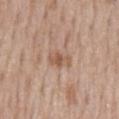Assessment: Recorded during total-body skin imaging; not selected for excision or biopsy. Image and clinical context: A male subject about 55 years old. The lesion-visualizer software estimated a footprint of about 4.5 mm², an outline eccentricity of about 0.85 (0 = round, 1 = elongated), and two-axis asymmetry of about 0.3. The software also gave a lesion color around L≈56 a*≈19 b*≈30 in CIELAB, roughly 9 lightness units darker than nearby skin, and a normalized lesion–skin contrast near 6.5. The analysis additionally found a border-irregularity index near 3/10 and peripheral color asymmetry of about 0.5. Cropped from a total-body skin-imaging series; the visible field is about 15 mm. The tile uses white-light illumination. On the mid back.